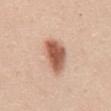Assessment: Captured during whole-body skin photography for melanoma surveillance; the lesion was not biopsied. Background: This image is a 15 mm lesion crop taken from a total-body photograph. Captured under white-light illumination. A male patient aged around 60. Located on the mid back.